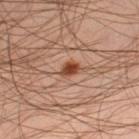Recorded during total-body skin imaging; not selected for excision or biopsy.
A 15 mm close-up extracted from a 3D total-body photography capture.
The tile uses cross-polarized illumination.
A male subject approximately 45 years of age.
Located on the left thigh.